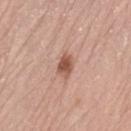Q: Was a biopsy performed?
A: imaged on a skin check; not biopsied
Q: What lighting was used for the tile?
A: white-light illumination
Q: What is the anatomic site?
A: the lower back
Q: How was this image acquired?
A: ~15 mm crop, total-body skin-cancer survey
Q: What are the patient's age and sex?
A: female, approximately 65 years of age